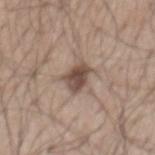<case>
<biopsy_status>not biopsied; imaged during a skin examination</biopsy_status>
<site>mid back</site>
<image>
  <source>total-body photography crop</source>
  <field_of_view_mm>15</field_of_view_mm>
</image>
<patient>
  <sex>male</sex>
  <age_approx>50</age_approx>
</patient>
</case>